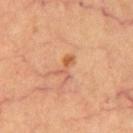| feature | finding |
|---|---|
| workup | catalogued during a skin exam; not biopsied |
| lighting | cross-polarized |
| lesion size | ~3 mm (longest diameter) |
| acquisition | total-body-photography crop, ~15 mm field of view |
| site | the mid back |
| image-analysis metrics | border irregularity of about 5.5 on a 0–10 scale, a within-lesion color-variation index near 0.5/10, and radial color variation of about 0 |
| subject | male, aged approximately 65 |